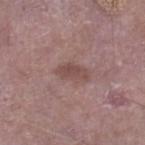| feature | finding |
|---|---|
| patient | male, aged 63 to 67 |
| size | about 3.5 mm |
| image | 15 mm crop, total-body photography |
| lighting | white-light illumination |
| location | the right lower leg |
| automated metrics | an area of roughly 5 mm², a shape eccentricity near 0.9, and a symmetry-axis asymmetry near 0.3; an average lesion color of about L≈47 a*≈19 b*≈21 (CIELAB), roughly 8 lightness units darker than nearby skin, and a normalized lesion–skin contrast near 6.5; a color-variation rating of about 1.5/10 and peripheral color asymmetry of about 0.5 |